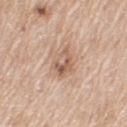Assessment:
The lesion was photographed on a routine skin check and not biopsied; there is no pathology result.